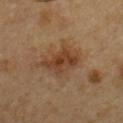Notes:
* follow-up — catalogued during a skin exam; not biopsied
* anatomic site — the chest
* image — ~15 mm crop, total-body skin-cancer survey
* patient — male, in their mid- to late 50s
* tile lighting — cross-polarized illumination
* diameter — ~5.5 mm (longest diameter)